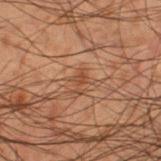Captured during whole-body skin photography for melanoma surveillance; the lesion was not biopsied. The lesion is located on the left thigh. The subject is a male roughly 50 years of age. Cropped from a whole-body photographic skin survey; the tile spans about 15 mm. The total-body-photography lesion software estimated a footprint of about 2.5 mm², a shape eccentricity near 0.85, and a symmetry-axis asymmetry near 0.45. The software also gave a border-irregularity rating of about 5.5/10 and a color-variation rating of about 0.5/10.